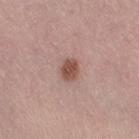Assessment:
The lesion was tiled from a total-body skin photograph and was not biopsied.
Acquisition and patient details:
Located on the right thigh. This is a white-light tile. The total-body-photography lesion software estimated an average lesion color of about L≈51 a*≈21 b*≈25 (CIELAB). Cropped from a total-body skin-imaging series; the visible field is about 15 mm. A female patient approximately 55 years of age. Measured at roughly 3 mm in maximum diameter.